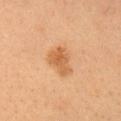Captured during whole-body skin photography for melanoma surveillance; the lesion was not biopsied.
A female subject in their 30s.
Imaged with cross-polarized lighting.
From the head or neck.
About 3.5 mm across.
Cropped from a whole-body photographic skin survey; the tile spans about 15 mm.